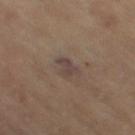biopsy status: imaged on a skin check; not biopsied | acquisition: 15 mm crop, total-body photography | subject: female, aged 68–72 | anatomic site: the right thigh.